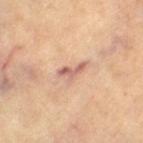Q: Was a biopsy performed?
A: no biopsy performed (imaged during a skin exam)
Q: How was the tile lit?
A: cross-polarized illumination
Q: Where on the body is the lesion?
A: the left thigh
Q: Who is the patient?
A: female, in their mid-60s
Q: What kind of image is this?
A: 15 mm crop, total-body photography
Q: Automated lesion metrics?
A: border irregularity of about 6 on a 0–10 scale and radial color variation of about 0
Q: How large is the lesion?
A: ~3.5 mm (longest diameter)